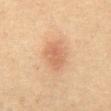The subject is a female approximately 50 years of age. Captured under cross-polarized illumination. On the abdomen. A close-up tile cropped from a whole-body skin photograph, about 15 mm across. An algorithmic analysis of the crop reported an area of roughly 8 mm², an outline eccentricity of about 0.65 (0 = round, 1 = elongated), and two-axis asymmetry of about 0.25. And it measured a lesion–skin lightness drop of about 8.